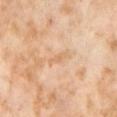{
  "site": "right thigh",
  "patient": {
    "sex": "female",
    "age_approx": 55
  },
  "image": {
    "source": "total-body photography crop",
    "field_of_view_mm": 15
  },
  "automated_metrics": {
    "cielab_L": 69,
    "cielab_a": 19,
    "cielab_b": 38,
    "vs_skin_darker_L": 7.0,
    "vs_skin_contrast_norm": 4.5
  },
  "lesion_size": {
    "long_diameter_mm_approx": 3.0
  }
}